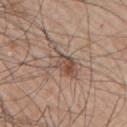| key | value |
|---|---|
| biopsy status | no biopsy performed (imaged during a skin exam) |
| imaging modality | ~15 mm crop, total-body skin-cancer survey |
| automated metrics | an average lesion color of about L≈51 a*≈16 b*≈25 (CIELAB) and a normalized lesion–skin contrast near 6.5; a classifier nevus-likeness of about 10/100 and lesion-presence confidence of about 100/100 |
| patient | male, aged approximately 65 |
| size | ≈5 mm |
| body site | the right upper arm |
| lighting | white-light |